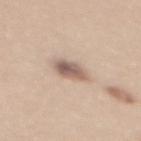| key | value |
|---|---|
| workup | total-body-photography surveillance lesion; no biopsy |
| image-analysis metrics | internal color variation of about 5.5 on a 0–10 scale and radial color variation of about 2; a nevus-likeness score of about 65/100 and a lesion-detection confidence of about 100/100 |
| image | ~15 mm crop, total-body skin-cancer survey |
| patient | female, roughly 35 years of age |
| site | the upper back |
| lesion diameter | about 3.5 mm |
| lighting | white-light illumination |